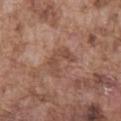Q: Was a biopsy performed?
A: no biopsy performed (imaged during a skin exam)
Q: Automated lesion metrics?
A: an outline eccentricity of about 0.8 (0 = round, 1 = elongated) and a shape-asymmetry score of about 0.5 (0 = symmetric); an average lesion color of about L≈48 a*≈20 b*≈27 (CIELAB) and a normalized lesion–skin contrast near 5.5
Q: How was this image acquired?
A: total-body-photography crop, ~15 mm field of view
Q: Where on the body is the lesion?
A: the front of the torso
Q: Lesion size?
A: ~4 mm (longest diameter)
Q: Illumination type?
A: white-light illumination
Q: Patient demographics?
A: male, aged 73 to 77